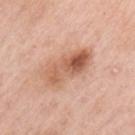Notes:
- biopsy status: imaged on a skin check; not biopsied
- subject: female, approximately 50 years of age
- tile lighting: white-light
- location: the left upper arm
- automated metrics: a border-irregularity rating of about 3/10 and a peripheral color-asymmetry measure near 4.5
- size: ≈6 mm
- image: ~15 mm crop, total-body skin-cancer survey